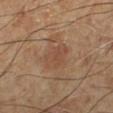Q: Was a biopsy performed?
A: imaged on a skin check; not biopsied
Q: How was this image acquired?
A: 15 mm crop, total-body photography
Q: What are the patient's age and sex?
A: male, aged around 70
Q: Automated lesion metrics?
A: a shape eccentricity near 0.7 and two-axis asymmetry of about 0.25
Q: Illumination type?
A: cross-polarized illumination
Q: How large is the lesion?
A: ≈3.5 mm
Q: Where on the body is the lesion?
A: the left lower leg